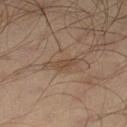Q: Is there a histopathology result?
A: total-body-photography surveillance lesion; no biopsy
Q: Patient demographics?
A: male, about 65 years old
Q: Where on the body is the lesion?
A: the left lower leg
Q: Illumination type?
A: cross-polarized
Q: What is the imaging modality?
A: total-body-photography crop, ~15 mm field of view